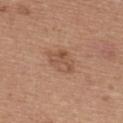  biopsy_status: not biopsied; imaged during a skin examination
  patient:
    sex: female
    age_approx: 35
  site: upper back
  image:
    source: total-body photography crop
    field_of_view_mm: 15